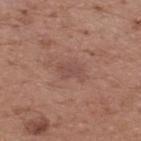The lesion was photographed on a routine skin check and not biopsied; there is no pathology result. Imaged with white-light lighting. The patient is a male aged approximately 65. The lesion is located on the back. Cropped from a total-body skin-imaging series; the visible field is about 15 mm.